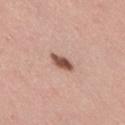workup = no biopsy performed (imaged during a skin exam); patient = female, in their mid- to late 30s; location = the right thigh; lighting = white-light; size = ≈3 mm; image source = 15 mm crop, total-body photography.